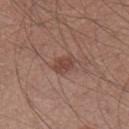Impression: No biopsy was performed on this lesion — it was imaged during a full skin examination and was not determined to be concerning. Acquisition and patient details: On the right thigh. A male subject roughly 45 years of age. A 15 mm close-up tile from a total-body photography series done for melanoma screening.